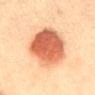Q: Is there a histopathology result?
A: catalogued during a skin exam; not biopsied
Q: What is the lesion's diameter?
A: ≈8 mm
Q: How was this image acquired?
A: ~15 mm tile from a whole-body skin photo
Q: What did automated image analysis measure?
A: a lesion area of about 29 mm², an eccentricity of roughly 0.8, and a shape-asymmetry score of about 0.15 (0 = symmetric); about 17 CIELAB-L* units darker than the surrounding skin and a normalized lesion–skin contrast near 10.5
Q: How was the tile lit?
A: cross-polarized
Q: What is the anatomic site?
A: the mid back
Q: What are the patient's age and sex?
A: male, aged 38–42